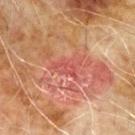Q: Was this lesion biopsied?
A: no biopsy performed (imaged during a skin exam)
Q: Patient demographics?
A: male, aged around 70
Q: What is the anatomic site?
A: the arm
Q: How was this image acquired?
A: 15 mm crop, total-body photography
Q: What lighting was used for the tile?
A: cross-polarized
Q: What did automated image analysis measure?
A: a footprint of about 5 mm², a shape eccentricity near 0.8, and a shape-asymmetry score of about 0.45 (0 = symmetric); an average lesion color of about L≈44 a*≈29 b*≈25 (CIELAB) and about 6 CIELAB-L* units darker than the surrounding skin; a border-irregularity index near 5/10, internal color variation of about 2 on a 0–10 scale, and peripheral color asymmetry of about 0.5; a detector confidence of about 80 out of 100 that the crop contains a lesion